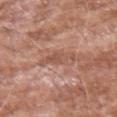- biopsy status — catalogued during a skin exam; not biopsied
- image — ~15 mm tile from a whole-body skin photo
- patient — male, about 60 years old
- body site — the left forearm
- image-analysis metrics — a border-irregularity rating of about 4/10, a within-lesion color-variation index near 2.5/10, and peripheral color asymmetry of about 1; a nevus-likeness score of about 0/100
- lighting — white-light illumination
- size — ≈4 mm